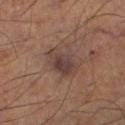Captured during whole-body skin photography for melanoma surveillance; the lesion was not biopsied. A region of skin cropped from a whole-body photographic capture, roughly 15 mm wide. Located on the right thigh. Approximately 4.5 mm at its widest. A male patient, in their mid- to late 50s. Imaged with cross-polarized lighting.